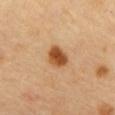Assessment: Imaged during a routine full-body skin examination; the lesion was not biopsied and no histopathology is available. Context: Imaged with cross-polarized lighting. A roughly 15 mm field-of-view crop from a total-body skin photograph. On the mid back. A female subject approximately 60 years of age. Longest diameter approximately 3.5 mm.